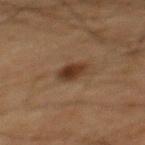This lesion was catalogued during total-body skin photography and was not selected for biopsy.
From the mid back.
Captured under cross-polarized illumination.
Cropped from a whole-body photographic skin survey; the tile spans about 15 mm.
About 3 mm across.
Automated tile analysis of the lesion measured an area of roughly 5 mm², a shape eccentricity near 0.7, and a shape-asymmetry score of about 0.25 (0 = symmetric). And it measured a lesion color around L≈28 a*≈16 b*≈24 in CIELAB, about 10 CIELAB-L* units darker than the surrounding skin, and a normalized border contrast of about 10. And it measured a border-irregularity index near 2/10, internal color variation of about 3 on a 0–10 scale, and radial color variation of about 1. The software also gave an automated nevus-likeness rating near 95 out of 100 and a detector confidence of about 100 out of 100 that the crop contains a lesion.
The patient is a male roughly 65 years of age.